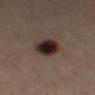Clinical impression: The lesion was tiled from a total-body skin photograph and was not biopsied. Background: Approximately 3.5 mm at its widest. This is a cross-polarized tile. A 15 mm close-up extracted from a 3D total-body photography capture. A male patient aged 63–67. On the left thigh.